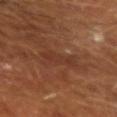Assessment:
Recorded during total-body skin imaging; not selected for excision or biopsy.
Acquisition and patient details:
Measured at roughly 4 mm in maximum diameter. Automated tile analysis of the lesion measured a symmetry-axis asymmetry near 0.3. It also reported a border-irregularity rating of about 4.5/10, a color-variation rating of about 1/10, and peripheral color asymmetry of about 0. On the left forearm. Captured under cross-polarized illumination. The patient is a male aged approximately 65. A 15 mm close-up extracted from a 3D total-body photography capture.